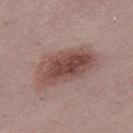notes=catalogued during a skin exam; not biopsied
lighting=white-light
patient=female, roughly 25 years of age
image source=~15 mm tile from a whole-body skin photo
body site=the leg
TBP lesion metrics=a mean CIELAB color near L≈48 a*≈20 b*≈22, about 12 CIELAB-L* units darker than the surrounding skin, and a normalized lesion–skin contrast near 9; a border-irregularity rating of about 2.5/10, a within-lesion color-variation index near 6.5/10, and radial color variation of about 2.5; a detector confidence of about 100 out of 100 that the crop contains a lesion